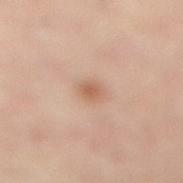  biopsy_status: not biopsied; imaged during a skin examination
  lesion_size:
    long_diameter_mm_approx: 2.0
  site: right lower leg
  image:
    source: total-body photography crop
    field_of_view_mm: 15
  patient:
    sex: male
    age_approx: 55
  lighting: cross-polarized
  automated_metrics:
    eccentricity: 0.3
    shape_asymmetry: 0.2
    cielab_L: 60
    cielab_a: 20
    cielab_b: 31
    vs_skin_darker_L: 9.0
    vs_skin_contrast_norm: 6.5
    border_irregularity_0_10: 1.5
    color_variation_0_10: 1.5